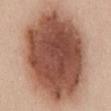Part of a total-body skin-imaging series; this lesion was reviewed on a skin check and was not flagged for biopsy.
Measured at roughly 13.5 mm in maximum diameter.
A female patient in their mid-40s.
Cropped from a whole-body photographic skin survey; the tile spans about 15 mm.
Located on the abdomen.
Imaged with white-light lighting.